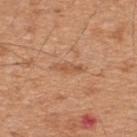• follow-up — catalogued during a skin exam; not biopsied
• image source — ~15 mm crop, total-body skin-cancer survey
• anatomic site — the upper back
• patient — male, aged 43 to 47
• lighting — white-light
• lesion diameter — about 3.5 mm